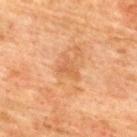Impression: This lesion was catalogued during total-body skin photography and was not selected for biopsy. Acquisition and patient details: Automated image analysis of the tile measured two-axis asymmetry of about 0.5. The analysis additionally found a mean CIELAB color near L≈50 a*≈22 b*≈35, roughly 6 lightness units darker than nearby skin, and a normalized lesion–skin contrast near 4.5. And it measured a within-lesion color-variation index near 1/10 and radial color variation of about 0.5. The software also gave a nevus-likeness score of about 0/100 and a lesion-detection confidence of about 100/100. Captured under cross-polarized illumination. A male patient, roughly 75 years of age. A 15 mm crop from a total-body photograph taken for skin-cancer surveillance. On the upper back. About 3 mm across.